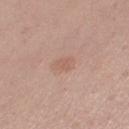Findings:
- notes · catalogued during a skin exam; not biopsied
- image · ~15 mm crop, total-body skin-cancer survey
- subject · female, aged around 30
- lesion diameter · ≈2.5 mm
- automated metrics · an area of roughly 4 mm² and a shape eccentricity near 0.7; a nevus-likeness score of about 0/100 and a detector confidence of about 100 out of 100 that the crop contains a lesion
- anatomic site · the right thigh
- lighting · white-light illumination